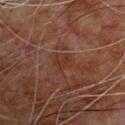* follow-up — imaged on a skin check; not biopsied
* size — ~2.5 mm (longest diameter)
* tile lighting — cross-polarized illumination
* patient — male, aged approximately 80
* image — ~15 mm crop, total-body skin-cancer survey
* body site — the front of the torso